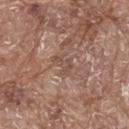Captured during whole-body skin photography for melanoma surveillance; the lesion was not biopsied.